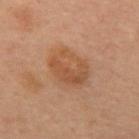The lesion was tiled from a total-body skin photograph and was not biopsied.
The lesion's longest dimension is about 5.5 mm.
The tile uses cross-polarized illumination.
The lesion is located on the chest.
Cropped from a whole-body photographic skin survey; the tile spans about 15 mm.
A male patient approximately 60 years of age.